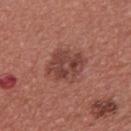Impression:
The lesion was photographed on a routine skin check and not biopsied; there is no pathology result.
Clinical summary:
Measured at roughly 5 mm in maximum diameter. The tile uses white-light illumination. The lesion-visualizer software estimated a nevus-likeness score of about 70/100 and a lesion-detection confidence of about 100/100. A lesion tile, about 15 mm wide, cut from a 3D total-body photograph. A male subject about 40 years old. The lesion is on the back.